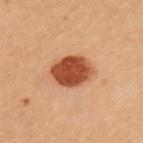Recorded during total-body skin imaging; not selected for excision or biopsy.
The patient is a female roughly 30 years of age.
A close-up tile cropped from a whole-body skin photograph, about 15 mm across.
Located on the upper back.